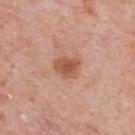Findings:
• notes — catalogued during a skin exam; not biopsied
• subject — male, roughly 75 years of age
• anatomic site — the upper back
• lesion diameter — ~3 mm (longest diameter)
• acquisition — 15 mm crop, total-body photography
• TBP lesion metrics — a classifier nevus-likeness of about 75/100 and a lesion-detection confidence of about 100/100
• tile lighting — white-light illumination